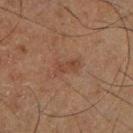follow-up: imaged on a skin check; not biopsied | automated metrics: a footprint of about 4 mm², an outline eccentricity of about 0.9 (0 = round, 1 = elongated), and two-axis asymmetry of about 0.35; a lesion color around L≈34 a*≈17 b*≈24 in CIELAB, roughly 5 lightness units darker than nearby skin, and a normalized border contrast of about 5 | lighting: cross-polarized | lesion diameter: ≈3 mm | subject: male, in their mid- to late 60s | imaging modality: ~15 mm crop, total-body skin-cancer survey | location: the right lower leg.